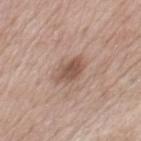Q: Was this lesion biopsied?
A: total-body-photography surveillance lesion; no biopsy